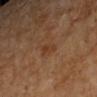Findings:
- follow-up · imaged on a skin check; not biopsied
- tile lighting · cross-polarized
- subject · female, aged 58 to 62
- image · total-body-photography crop, ~15 mm field of view
- anatomic site · the left upper arm
- image-analysis metrics · an area of roughly 2.5 mm² and a symmetry-axis asymmetry near 0.4; about 6 CIELAB-L* units darker than the surrounding skin and a normalized border contrast of about 6; a nevus-likeness score of about 0/100 and a lesion-detection confidence of about 100/100